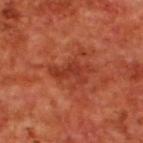No biopsy was performed on this lesion — it was imaged during a full skin examination and was not determined to be concerning. Measured at roughly 4.5 mm in maximum diameter. The patient is a male roughly 70 years of age. Cropped from a total-body skin-imaging series; the visible field is about 15 mm. The lesion is located on the upper back.